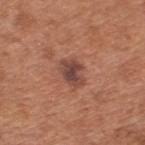Imaged during a routine full-body skin examination; the lesion was not biopsied and no histopathology is available.
A region of skin cropped from a whole-body photographic capture, roughly 15 mm wide.
On the upper back.
Approximately 3.5 mm at its widest.
Captured under white-light illumination.
The lesion-visualizer software estimated a footprint of about 7 mm², an eccentricity of roughly 0.75, and a symmetry-axis asymmetry near 0.2. And it measured an average lesion color of about L≈45 a*≈23 b*≈26 (CIELAB), about 11 CIELAB-L* units darker than the surrounding skin, and a normalized lesion–skin contrast near 9.
A male patient in their mid- to late 60s.